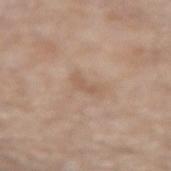biopsy status — no biopsy performed (imaged during a skin exam)